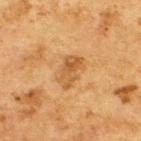Impression: The lesion was tiled from a total-body skin photograph and was not biopsied. Background: A region of skin cropped from a whole-body photographic capture, roughly 15 mm wide. This is a cross-polarized tile. The recorded lesion diameter is about 4 mm. A male patient, approximately 75 years of age. On the upper back. Automated image analysis of the tile measured a footprint of about 7 mm² and two-axis asymmetry of about 0.25. The software also gave roughly 8 lightness units darker than nearby skin and a normalized border contrast of about 6.5.